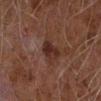This lesion was catalogued during total-body skin photography and was not selected for biopsy. A roughly 15 mm field-of-view crop from a total-body skin photograph. The tile uses cross-polarized illumination. From the left forearm. A male subject aged 58–62.